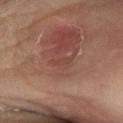The lesion was photographed on a routine skin check and not biopsied; there is no pathology result. Approximately 2.5 mm at its widest. A close-up tile cropped from a whole-body skin photograph, about 15 mm across. A female patient approximately 50 years of age. Located on the left lower leg. The lesion-visualizer software estimated an area of roughly 2 mm² and an outline eccentricity of about 0.95 (0 = round, 1 = elongated). It also reported a mean CIELAB color near L≈42 a*≈23 b*≈25, roughly 5 lightness units darker than nearby skin, and a normalized border contrast of about 4.5. The software also gave internal color variation of about 0 on a 0–10 scale and radial color variation of about 0.